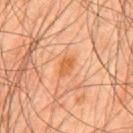Q: Is there a histopathology result?
A: no biopsy performed (imaged during a skin exam)
Q: How was this image acquired?
A: ~15 mm crop, total-body skin-cancer survey
Q: Where on the body is the lesion?
A: the mid back
Q: Who is the patient?
A: male, about 45 years old
Q: Illumination type?
A: cross-polarized
Q: What is the lesion's diameter?
A: ≈3 mm